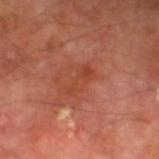Case summary:
• workup: total-body-photography surveillance lesion; no biopsy